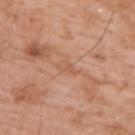Captured during whole-body skin photography for melanoma surveillance; the lesion was not biopsied. The lesion is located on the upper back. Imaged with white-light lighting. Cropped from a whole-body photographic skin survey; the tile spans about 15 mm. The patient is a male aged around 60.